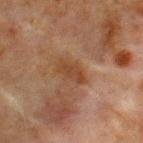Assessment:
Imaged during a routine full-body skin examination; the lesion was not biopsied and no histopathology is available.
Context:
Imaged with cross-polarized lighting. A region of skin cropped from a whole-body photographic capture, roughly 15 mm wide. Automated tile analysis of the lesion measured an area of roughly 6 mm² and a shape eccentricity near 0.85. Located on the chest. Measured at roughly 3.5 mm in maximum diameter. The patient is a male aged approximately 65.